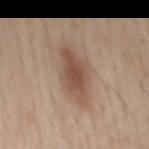The lesion was photographed on a routine skin check and not biopsied; there is no pathology result. Imaged with white-light lighting. Longest diameter approximately 6.5 mm. Cropped from a whole-body photographic skin survey; the tile spans about 15 mm. The patient is a male in their 60s. From the mid back.